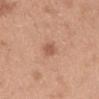Captured during whole-body skin photography for melanoma surveillance; the lesion was not biopsied.
A male subject about 50 years old.
A 15 mm close-up tile from a total-body photography series done for melanoma screening.
The tile uses white-light illumination.
The lesion is located on the front of the torso.